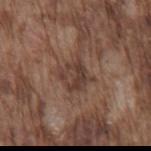workup — catalogued during a skin exam; not biopsied
automated metrics — a footprint of about 9 mm² and an eccentricity of roughly 0.3; a border-irregularity rating of about 3.5/10, a color-variation rating of about 5/10, and a peripheral color-asymmetry measure near 2
site — the mid back
acquisition — 15 mm crop, total-body photography
tile lighting — white-light
patient — male, aged approximately 75